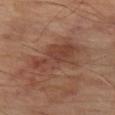The lesion was photographed on a routine skin check and not biopsied; there is no pathology result.
A male subject aged around 70.
From the left thigh.
Cropped from a whole-body photographic skin survey; the tile spans about 15 mm.